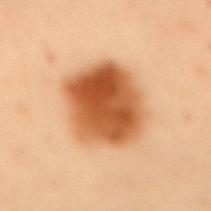Assessment: The lesion was tiled from a total-body skin photograph and was not biopsied. Background: Located on the mid back. Captured under cross-polarized illumination. A female subject, aged approximately 50. Cropped from a whole-body photographic skin survey; the tile spans about 15 mm. The lesion's longest dimension is about 6.5 mm.